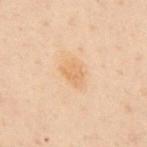Impression:
Recorded during total-body skin imaging; not selected for excision or biopsy.
Image and clinical context:
The tile uses cross-polarized illumination. The lesion is on the mid back. The patient is a male aged 48–52. A roughly 15 mm field-of-view crop from a total-body skin photograph. Approximately 3 mm at its widest.